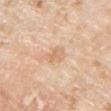Clinical impression:
Imaged during a routine full-body skin examination; the lesion was not biopsied and no histopathology is available.
Background:
The tile uses white-light illumination. The recorded lesion diameter is about 2.5 mm. An algorithmic analysis of the crop reported an average lesion color of about L≈69 a*≈19 b*≈35 (CIELAB), a lesion–skin lightness drop of about 8, and a normalized lesion–skin contrast near 5.5. It also reported a border-irregularity index near 4/10, a within-lesion color-variation index near 1.5/10, and a peripheral color-asymmetry measure near 0.5. The software also gave an automated nevus-likeness rating near 0 out of 100 and a lesion-detection confidence of about 100/100. The subject is a male approximately 75 years of age. From the right upper arm. Cropped from a whole-body photographic skin survey; the tile spans about 15 mm.